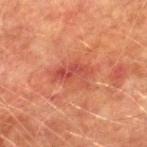Q: Is there a histopathology result?
A: imaged on a skin check; not biopsied
Q: How was this image acquired?
A: total-body-photography crop, ~15 mm field of view
Q: What is the anatomic site?
A: the right lower leg
Q: What are the patient's age and sex?
A: male, aged 73 to 77